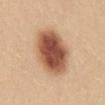Q: Was this lesion biopsied?
A: imaged on a skin check; not biopsied
Q: What did automated image analysis measure?
A: a border-irregularity rating of about 1/10 and peripheral color asymmetry of about 2; a classifier nevus-likeness of about 100/100 and lesion-presence confidence of about 100/100
Q: What is the lesion's diameter?
A: about 6 mm
Q: How was this image acquired?
A: ~15 mm crop, total-body skin-cancer survey
Q: Who is the patient?
A: female, in their mid-20s
Q: Illumination type?
A: white-light
Q: What is the anatomic site?
A: the mid back